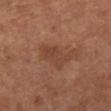Impression: Recorded during total-body skin imaging; not selected for excision or biopsy. Clinical summary: A region of skin cropped from a whole-body photographic capture, roughly 15 mm wide. The lesion is located on the left lower leg. About 4 mm across. A male subject, about 65 years old. Captured under cross-polarized illumination. Automated image analysis of the tile measured a mean CIELAB color near L≈41 a*≈22 b*≈29 and about 7 CIELAB-L* units darker than the surrounding skin. And it measured border irregularity of about 5.5 on a 0–10 scale and a color-variation rating of about 1/10.